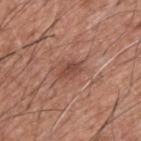Impression:
This lesion was catalogued during total-body skin photography and was not selected for biopsy.
Image and clinical context:
Captured under white-light illumination. Cropped from a whole-body photographic skin survey; the tile spans about 15 mm. On the upper back. A male subject, aged 58 to 62. Longest diameter approximately 3 mm.